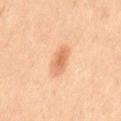Recorded during total-body skin imaging; not selected for excision or biopsy. The total-body-photography lesion software estimated about 9 CIELAB-L* units darker than the surrounding skin and a normalized lesion–skin contrast near 6.5. And it measured a border-irregularity index near 2/10, a within-lesion color-variation index near 3/10, and radial color variation of about 0.5. The analysis additionally found an automated nevus-likeness rating near 90 out of 100 and a lesion-detection confidence of about 100/100. Measured at roughly 3.5 mm in maximum diameter. Imaged with cross-polarized lighting. From the lower back. A female subject aged 18–22. This image is a 15 mm lesion crop taken from a total-body photograph.